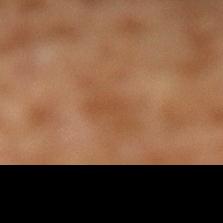Clinical impression: The lesion was photographed on a routine skin check and not biopsied; there is no pathology result.